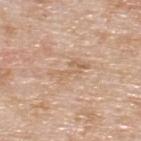Located on the upper back. This image is a 15 mm lesion crop taken from a total-body photograph. Captured under white-light illumination. Automated tile analysis of the lesion measured an average lesion color of about L≈63 a*≈17 b*≈32 (CIELAB), roughly 7 lightness units darker than nearby skin, and a normalized lesion–skin contrast near 5. A male subject, approximately 60 years of age. Approximately 5 mm at its widest.